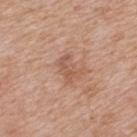biopsy status = total-body-photography surveillance lesion; no biopsy | automated metrics = a border-irregularity rating of about 6/10 and radial color variation of about 0; an automated nevus-likeness rating near 0 out of 100 and lesion-presence confidence of about 100/100 | acquisition = ~15 mm tile from a whole-body skin photo | site = the mid back | lesion diameter = ~2.5 mm (longest diameter) | tile lighting = white-light | subject = male, in their 60s.